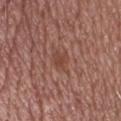Recorded during total-body skin imaging; not selected for excision or biopsy.
Located on the head or neck.
A lesion tile, about 15 mm wide, cut from a 3D total-body photograph.
A male subject aged approximately 55.